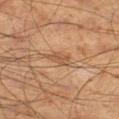follow-up: imaged on a skin check; not biopsied.